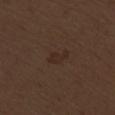{
  "biopsy_status": "not biopsied; imaged during a skin examination",
  "lesion_size": {
    "long_diameter_mm_approx": 3.0
  },
  "site": "right thigh",
  "patient": {
    "sex": "male",
    "age_approx": 70
  },
  "lighting": "white-light",
  "image": {
    "source": "total-body photography crop",
    "field_of_view_mm": 15
  }
}